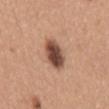Imaged during a routine full-body skin examination; the lesion was not biopsied and no histopathology is available.
The lesion's longest dimension is about 5 mm.
Captured under white-light illumination.
The lesion is located on the mid back.
The patient is a female aged approximately 30.
A 15 mm close-up tile from a total-body photography series done for melanoma screening.